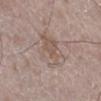workup: total-body-photography surveillance lesion; no biopsy | anatomic site: the right lower leg | size: ≈5 mm | acquisition: 15 mm crop, total-body photography | patient: male, aged around 50 | automated lesion analysis: a nevus-likeness score of about 0/100 and a lesion-detection confidence of about 100/100.